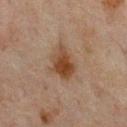Imaged during a routine full-body skin examination; the lesion was not biopsied and no histopathology is available. The lesion-visualizer software estimated a lesion color around L≈35 a*≈15 b*≈26 in CIELAB. The analysis additionally found border irregularity of about 3 on a 0–10 scale, internal color variation of about 5 on a 0–10 scale, and a peripheral color-asymmetry measure near 1.5. The analysis additionally found a classifier nevus-likeness of about 95/100 and a lesion-detection confidence of about 100/100. The lesion is located on the mid back. A male patient aged approximately 65. The tile uses cross-polarized illumination. Cropped from a whole-body photographic skin survey; the tile spans about 15 mm.